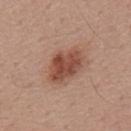Q: Is there a histopathology result?
A: catalogued during a skin exam; not biopsied
Q: How was this image acquired?
A: ~15 mm crop, total-body skin-cancer survey
Q: Lesion location?
A: the back
Q: How large is the lesion?
A: ~5 mm (longest diameter)
Q: Who is the patient?
A: male, approximately 55 years of age